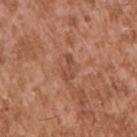Case summary:
• notes: imaged on a skin check; not biopsied
• body site: the right upper arm
• automated lesion analysis: a lesion color around L≈48 a*≈24 b*≈31 in CIELAB; a border-irregularity rating of about 4.5/10, a within-lesion color-variation index near 1/10, and a peripheral color-asymmetry measure near 0.5
• tile lighting: white-light illumination
• image: ~15 mm tile from a whole-body skin photo
• patient: male, roughly 45 years of age